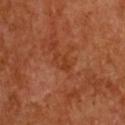notes: no biopsy performed (imaged during a skin exam) | subject: female, aged around 65 | location: the chest | acquisition: total-body-photography crop, ~15 mm field of view | size: about 2.5 mm.